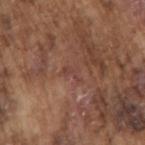Recorded during total-body skin imaging; not selected for excision or biopsy.